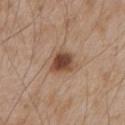No biopsy was performed on this lesion — it was imaged during a full skin examination and was not determined to be concerning.
Cropped from a total-body skin-imaging series; the visible field is about 15 mm.
On the chest.
A male patient, approximately 65 years of age.